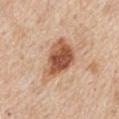notes=imaged on a skin check; not biopsied | patient=male, approximately 55 years of age | illumination=white-light | lesion size=about 6 mm | TBP lesion metrics=an average lesion color of about L≈56 a*≈23 b*≈33 (CIELAB), about 15 CIELAB-L* units darker than the surrounding skin, and a normalized border contrast of about 10; a lesion-detection confidence of about 100/100 | site=the mid back | acquisition=15 mm crop, total-body photography.